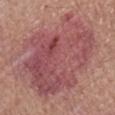follow-up: imaged on a skin check; not biopsied | size: about 11 mm | imaging modality: 15 mm crop, total-body photography | automated lesion analysis: an average lesion color of about L≈50 a*≈27 b*≈20 (CIELAB), roughly 9 lightness units darker than nearby skin, and a lesion-to-skin contrast of about 7.5 (normalized; higher = more distinct); a border-irregularity index near 4.5/10, internal color variation of about 6.5 on a 0–10 scale, and radial color variation of about 2.5; a detector confidence of about 100 out of 100 that the crop contains a lesion | illumination: white-light illumination | site: the left forearm | subject: female, aged 58–62.